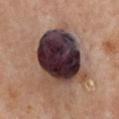Notes:
* biopsy status · catalogued during a skin exam; not biopsied
* lesion diameter · ~8 mm (longest diameter)
* TBP lesion metrics · a lesion color around L≈33 a*≈17 b*≈15 in CIELAB, about 25 CIELAB-L* units darker than the surrounding skin, and a normalized lesion–skin contrast near 20.5; a nevus-likeness score of about 0/100 and a detector confidence of about 100 out of 100 that the crop contains a lesion
* subject · female, aged approximately 60
* location · the front of the torso
* imaging modality · 15 mm crop, total-body photography
* tile lighting · cross-polarized